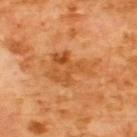<case>
  <biopsy_status>not biopsied; imaged during a skin examination</biopsy_status>
  <patient>
    <sex>male</sex>
    <age_approx>65</age_approx>
  </patient>
  <site>upper back</site>
  <lesion_size>
    <long_diameter_mm_approx>5.0</long_diameter_mm_approx>
  </lesion_size>
  <lighting>cross-polarized</lighting>
  <image>
    <source>total-body photography crop</source>
    <field_of_view_mm>15</field_of_view_mm>
  </image>
</case>